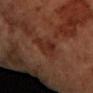Impression: Recorded during total-body skin imaging; not selected for excision or biopsy. Context: A female subject, aged approximately 70. About 4 mm across. Cropped from a total-body skin-imaging series; the visible field is about 15 mm. On the arm. Automated image analysis of the tile measured a border-irregularity index near 4/10, a within-lesion color-variation index near 2.5/10, and radial color variation of about 1. And it measured lesion-presence confidence of about 100/100. This is a cross-polarized tile.